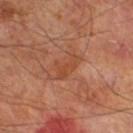<record>
<biopsy_status>not biopsied; imaged during a skin examination</biopsy_status>
<patient>
  <sex>male</sex>
  <age_approx>70</age_approx>
</patient>
<image>
  <source>total-body photography crop</source>
  <field_of_view_mm>15</field_of_view_mm>
</image>
<lesion_size>
  <long_diameter_mm_approx>4.0</long_diameter_mm_approx>
</lesion_size>
<lighting>cross-polarized</lighting>
<site>leg</site>
<automated_metrics>
  <area_mm2_approx>5.5</area_mm2_approx>
  <eccentricity>0.85</eccentricity>
  <shape_asymmetry>0.55</shape_asymmetry>
  <nevus_likeness_0_100>0</nevus_likeness_0_100>
  <lesion_detection_confidence_0_100>100</lesion_detection_confidence_0_100>
</automated_metrics>
</record>